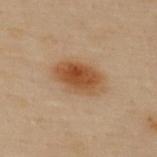The lesion-visualizer software estimated a lesion-to-skin contrast of about 9.5 (normalized; higher = more distinct). The analysis additionally found a classifier nevus-likeness of about 100/100 and a detector confidence of about 100 out of 100 that the crop contains a lesion.
From the upper back.
Imaged with cross-polarized lighting.
The patient is a female aged 58 to 62.
About 5.5 mm across.
A roughly 15 mm field-of-view crop from a total-body skin photograph.